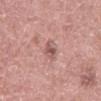Captured during whole-body skin photography for melanoma surveillance; the lesion was not biopsied.
From the abdomen.
A 15 mm close-up extracted from a 3D total-body photography capture.
A male subject in their 60s.